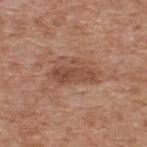| key | value |
|---|---|
| biopsy status | no biopsy performed (imaged during a skin exam) |
| lighting | white-light |
| patient | male, in their 60s |
| image source | ~15 mm crop, total-body skin-cancer survey |
| body site | the upper back |